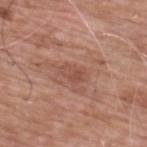Impression: Imaged during a routine full-body skin examination; the lesion was not biopsied and no histopathology is available. Image and clinical context: This is a white-light tile. The lesion is located on the back. Automated tile analysis of the lesion measured a footprint of about 4.5 mm² and a symmetry-axis asymmetry near 0.25. The analysis additionally found a lesion color around L≈50 a*≈23 b*≈27 in CIELAB, about 7 CIELAB-L* units darker than the surrounding skin, and a normalized lesion–skin contrast near 5. Approximately 3 mm at its widest. A 15 mm close-up tile from a total-body photography series done for melanoma screening. A male patient aged approximately 60.